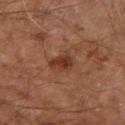Assessment: Captured during whole-body skin photography for melanoma surveillance; the lesion was not biopsied. Background: On the right leg. Measured at roughly 3 mm in maximum diameter. A 15 mm close-up extracted from a 3D total-body photography capture. Automated image analysis of the tile measured an outline eccentricity of about 0.7 (0 = round, 1 = elongated). It also reported an average lesion color of about L≈35 a*≈23 b*≈28 (CIELAB), a lesion–skin lightness drop of about 9, and a normalized border contrast of about 7.5. The software also gave border irregularity of about 3.5 on a 0–10 scale and radial color variation of about 1. A male subject, about 60 years old. The tile uses cross-polarized illumination.